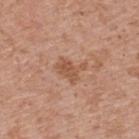| field | value |
|---|---|
| lesion size | ≈3.5 mm |
| illumination | white-light illumination |
| body site | the upper back |
| automated lesion analysis | border irregularity of about 4.5 on a 0–10 scale, a color-variation rating of about 2/10, and a peripheral color-asymmetry measure near 1; a nevus-likeness score of about 0/100 |
| image source | ~15 mm crop, total-body skin-cancer survey |
| patient | male, aged 68–72 |